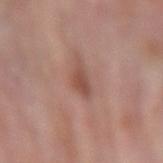{"biopsy_status": "not biopsied; imaged during a skin examination", "lighting": "white-light", "patient": {"sex": "male", "age_approx": 75}, "site": "right forearm", "image": {"source": "total-body photography crop", "field_of_view_mm": 15}, "automated_metrics": {"border_irregularity_0_10": 3.0, "color_variation_0_10": 2.0}, "lesion_size": {"long_diameter_mm_approx": 3.0}}